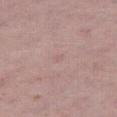{"biopsy_status": "not biopsied; imaged during a skin examination", "patient": {"sex": "female", "age_approx": 60}, "site": "leg", "lighting": "white-light", "image": {"source": "total-body photography crop", "field_of_view_mm": 15}}